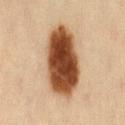| field | value |
|---|---|
| notes | imaged on a skin check; not biopsied |
| patient | female, in their mid- to late 40s |
| diameter | about 8 mm |
| site | the lower back |
| image source | total-body-photography crop, ~15 mm field of view |